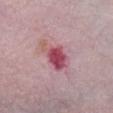illumination: white-light illumination | subject: female, aged approximately 40 | diameter: about 4.5 mm | body site: the chest | image: ~15 mm crop, total-body skin-cancer survey | TBP lesion metrics: a footprint of about 9 mm² and a shape-asymmetry score of about 0.3 (0 = symmetric); border irregularity of about 4 on a 0–10 scale, a color-variation rating of about 4.5/10, and radial color variation of about 1.5; lesion-presence confidence of about 100/100.